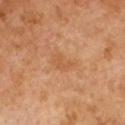| field | value |
|---|---|
| notes | total-body-photography surveillance lesion; no biopsy |
| automated lesion analysis | a mean CIELAB color near L≈55 a*≈23 b*≈38, roughly 6 lightness units darker than nearby skin, and a normalized lesion–skin contrast near 5; a detector confidence of about 100 out of 100 that the crop contains a lesion |
| image | 15 mm crop, total-body photography |
| lesion size | ≈3 mm |
| patient | male, approximately 65 years of age |
| lighting | cross-polarized |